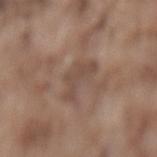Assessment:
Recorded during total-body skin imaging; not selected for excision or biopsy.